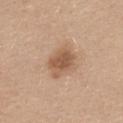Q: Was this lesion biopsied?
A: imaged on a skin check; not biopsied
Q: How large is the lesion?
A: about 4 mm
Q: What lighting was used for the tile?
A: white-light illumination
Q: Lesion location?
A: the upper back
Q: What are the patient's age and sex?
A: female, in their 30s
Q: What is the imaging modality?
A: total-body-photography crop, ~15 mm field of view